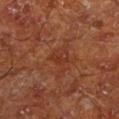A male subject aged around 65.
On the left lower leg.
Cropped from a total-body skin-imaging series; the visible field is about 15 mm.
Measured at roughly 2.5 mm in maximum diameter.